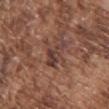Impression: Captured during whole-body skin photography for melanoma surveillance; the lesion was not biopsied. Clinical summary: The lesion is located on the front of the torso. The tile uses white-light illumination. Cropped from a whole-body photographic skin survey; the tile spans about 15 mm. The lesion's longest dimension is about 3.5 mm. A male patient, about 75 years old.